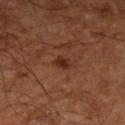Imaged during a routine full-body skin examination; the lesion was not biopsied and no histopathology is available.
A close-up tile cropped from a whole-body skin photograph, about 15 mm across.
The lesion is on the left lower leg.
The subject is aged 63 to 67.